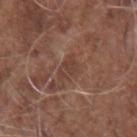Clinical impression: No biopsy was performed on this lesion — it was imaged during a full skin examination and was not determined to be concerning. Clinical summary: This image is a 15 mm lesion crop taken from a total-body photograph. The lesion-visualizer software estimated a footprint of about 4.5 mm², an outline eccentricity of about 0.75 (0 = round, 1 = elongated), and a symmetry-axis asymmetry near 0.3. And it measured about 7 CIELAB-L* units darker than the surrounding skin and a normalized border contrast of about 5.5. The analysis additionally found border irregularity of about 3 on a 0–10 scale and a peripheral color-asymmetry measure near 0.5. The analysis additionally found lesion-presence confidence of about 85/100. The tile uses white-light illumination. A male patient, aged 73 to 77. The lesion's longest dimension is about 3 mm. The lesion is located on the right forearm.